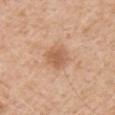• workup · catalogued during a skin exam; not biopsied
• subject · male, aged approximately 60
• image · ~15 mm crop, total-body skin-cancer survey
• TBP lesion metrics · a border-irregularity rating of about 1.5/10 and a color-variation rating of about 2.5/10; an automated nevus-likeness rating near 10 out of 100
• size · ≈3 mm
• location · the right upper arm
• illumination · white-light illumination